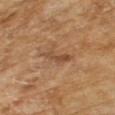diameter: about 3.5 mm | automated lesion analysis: a normalized border contrast of about 6.5 | tile lighting: cross-polarized illumination | image: ~15 mm crop, total-body skin-cancer survey | patient: male, aged 63 to 67.